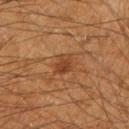image source: ~15 mm crop, total-body skin-cancer survey
patient: male, in their 60s
diameter: about 2.5 mm
image-analysis metrics: a footprint of about 4.5 mm², an outline eccentricity of about 0.7 (0 = round, 1 = elongated), and two-axis asymmetry of about 0.2; a lesion color around L≈38 a*≈22 b*≈33 in CIELAB and a lesion-to-skin contrast of about 7 (normalized; higher = more distinct); border irregularity of about 2.5 on a 0–10 scale, a within-lesion color-variation index near 3/10, and a peripheral color-asymmetry measure near 1; a detector confidence of about 100 out of 100 that the crop contains a lesion
tile lighting: cross-polarized illumination
body site: the right forearm